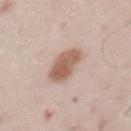| key | value |
|---|---|
| notes | no biopsy performed (imaged during a skin exam) |
| body site | the front of the torso |
| patient | male, in their 60s |
| imaging modality | total-body-photography crop, ~15 mm field of view |